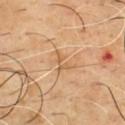<record>
  <patient>
    <sex>male</sex>
    <age_approx>50</age_approx>
  </patient>
  <site>front of the torso</site>
  <automated_metrics>
    <cielab_L>60</cielab_L>
    <cielab_a>20</cielab_a>
    <cielab_b>38</cielab_b>
    <vs_skin_darker_L>8.0</vs_skin_darker_L>
    <vs_skin_contrast_norm>5.5</vs_skin_contrast_norm>
    <border_irregularity_0_10>7.0</border_irregularity_0_10>
    <color_variation_0_10>0.0</color_variation_0_10>
    <peripheral_color_asymmetry>0.0</peripheral_color_asymmetry>
    <lesion_detection_confidence_0_100>95</lesion_detection_confidence_0_100>
  </automated_metrics>
  <lighting>cross-polarized</lighting>
  <image>
    <source>total-body photography crop</source>
    <field_of_view_mm>15</field_of_view_mm>
  </image>
</record>